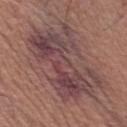Imaged during a routine full-body skin examination; the lesion was not biopsied and no histopathology is available.
The lesion-visualizer software estimated border irregularity of about 7.5 on a 0–10 scale. It also reported an automated nevus-likeness rating near 0 out of 100.
Located on the left upper arm.
A male subject, in their mid-50s.
Approximately 10 mm at its widest.
This image is a 15 mm lesion crop taken from a total-body photograph.
Imaged with white-light lighting.